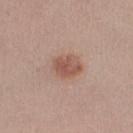A roughly 15 mm field-of-view crop from a total-body skin photograph.
The lesion is located on the right lower leg.
The subject is a female aged around 25.
Approximately 3.5 mm at its widest.